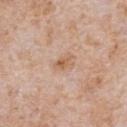follow-up — catalogued during a skin exam; not biopsied